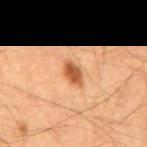biopsy status: catalogued during a skin exam; not biopsied
diameter: ~3 mm (longest diameter)
image: ~15 mm crop, total-body skin-cancer survey
illumination: cross-polarized illumination
site: the mid back
automated lesion analysis: a lesion area of about 5 mm², an eccentricity of roughly 0.8, and a shape-asymmetry score of about 0.2 (0 = symmetric); about 12 CIELAB-L* units darker than the surrounding skin and a normalized lesion–skin contrast near 9; a border-irregularity index near 2/10, a color-variation rating of about 2.5/10, and peripheral color asymmetry of about 1; a classifier nevus-likeness of about 95/100 and lesion-presence confidence of about 100/100
patient: male, aged approximately 65